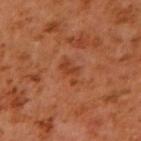This lesion was catalogued during total-body skin photography and was not selected for biopsy.
A 15 mm close-up tile from a total-body photography series done for melanoma screening.
The total-body-photography lesion software estimated a lesion area of about 4 mm², an outline eccentricity of about 0.85 (0 = round, 1 = elongated), and two-axis asymmetry of about 0.4. The analysis additionally found a lesion-detection confidence of about 100/100.
A male patient in their 60s.
Captured under cross-polarized illumination.
From the right upper arm.
Approximately 3.5 mm at its widest.